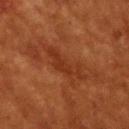This lesion was catalogued during total-body skin photography and was not selected for biopsy. A 15 mm crop from a total-body photograph taken for skin-cancer surveillance. Captured under cross-polarized illumination. The recorded lesion diameter is about 5.5 mm. On the upper back. The patient is a female approximately 80 years of age.